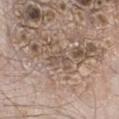Imaged during a routine full-body skin examination; the lesion was not biopsied and no histopathology is available. Captured under white-light illumination. A 15 mm close-up tile from a total-body photography series done for melanoma screening. Located on the right lower leg. The recorded lesion diameter is about 3 mm. An algorithmic analysis of the crop reported border irregularity of about 6.5 on a 0–10 scale, internal color variation of about 0 on a 0–10 scale, and radial color variation of about 0. And it measured an automated nevus-likeness rating near 0 out of 100 and a detector confidence of about 0 out of 100 that the crop contains a lesion. A male patient, roughly 60 years of age.